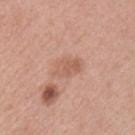The patient is a male approximately 60 years of age. Imaged with white-light lighting. Cropped from a total-body skin-imaging series; the visible field is about 15 mm. On the chest. Automated image analysis of the tile measured an automated nevus-likeness rating near 0 out of 100 and a detector confidence of about 100 out of 100 that the crop contains a lesion.